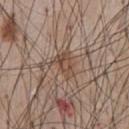notes: total-body-photography surveillance lesion; no biopsy | size: ~3 mm (longest diameter) | image source: ~15 mm tile from a whole-body skin photo | body site: the chest | subject: male, aged around 60.